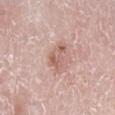workup — total-body-photography surveillance lesion; no biopsy | image source — ~15 mm crop, total-body skin-cancer survey | subject — male, approximately 80 years of age | tile lighting — white-light | location — the right lower leg.